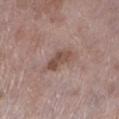{"biopsy_status": "not biopsied; imaged during a skin examination", "patient": {"sex": "female", "age_approx": 70}, "image": {"source": "total-body photography crop", "field_of_view_mm": 15}, "site": "left lower leg", "lighting": "white-light"}